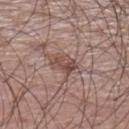Imaged during a routine full-body skin examination; the lesion was not biopsied and no histopathology is available. The patient is a male about 60 years old. A 15 mm close-up tile from a total-body photography series done for melanoma screening. From the left lower leg. This is a white-light tile. The lesion-visualizer software estimated a within-lesion color-variation index near 5/10. The software also gave lesion-presence confidence of about 100/100. Longest diameter approximately 3.5 mm.